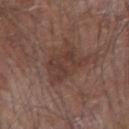Captured during whole-body skin photography for melanoma surveillance; the lesion was not biopsied. Cropped from a whole-body photographic skin survey; the tile spans about 15 mm. The lesion is on the arm. A male subject aged approximately 80. The tile uses white-light illumination. Measured at roughly 5 mm in maximum diameter.